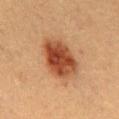Acquisition and patient details: The patient is a male approximately 50 years of age. Captured under cross-polarized illumination. The recorded lesion diameter is about 5.5 mm. A 15 mm crop from a total-body photograph taken for skin-cancer surveillance. The lesion is located on the mid back.